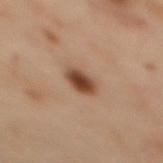workup=imaged on a skin check; not biopsied
imaging modality=15 mm crop, total-body photography
lighting=cross-polarized
size=~3 mm (longest diameter)
body site=the upper back
patient=female, aged approximately 55
image-analysis metrics=roughly 13 lightness units darker than nearby skin; a border-irregularity index near 1/10 and peripheral color asymmetry of about 1; a classifier nevus-likeness of about 100/100 and a lesion-detection confidence of about 100/100